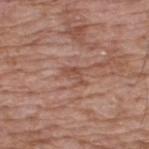{
  "image": {
    "source": "total-body photography crop",
    "field_of_view_mm": 15
  },
  "patient": {
    "sex": "male",
    "age_approx": 60
  },
  "lesion_size": {
    "long_diameter_mm_approx": 3.0
  },
  "site": "upper back",
  "lighting": "white-light"
}